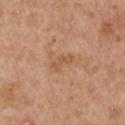{
  "biopsy_status": "not biopsied; imaged during a skin examination",
  "lesion_size": {
    "long_diameter_mm_approx": 3.0
  },
  "image": {
    "source": "total-body photography crop",
    "field_of_view_mm": 15
  },
  "site": "right upper arm",
  "patient": {
    "sex": "male",
    "age_approx": 55
  },
  "lighting": "white-light"
}